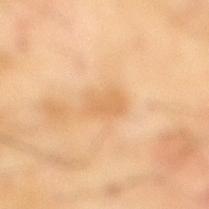Imaged during a routine full-body skin examination; the lesion was not biopsied and no histopathology is available.
Cropped from a total-body skin-imaging series; the visible field is about 15 mm.
Longest diameter approximately 4 mm.
A male subject aged approximately 70.
The tile uses cross-polarized illumination.
From the right lower leg.